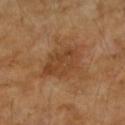Impression:
Imaged during a routine full-body skin examination; the lesion was not biopsied and no histopathology is available.
Context:
The lesion-visualizer software estimated an average lesion color of about L≈44 a*≈22 b*≈35 (CIELAB), roughly 8 lightness units darker than nearby skin, and a lesion-to-skin contrast of about 6.5 (normalized; higher = more distinct). Cropped from a whole-body photographic skin survey; the tile spans about 15 mm. Captured under cross-polarized illumination. From the right forearm. The subject is a female about 70 years old.